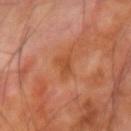Case summary:
- subject · male, aged 68–72
- imaging modality · ~15 mm crop, total-body skin-cancer survey
- lighting · cross-polarized illumination
- location · the right forearm
- diameter · ~2.5 mm (longest diameter)